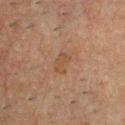On the chest.
A male subject approximately 50 years of age.
The recorded lesion diameter is about 2.5 mm.
A lesion tile, about 15 mm wide, cut from a 3D total-body photograph.
The tile uses cross-polarized illumination.